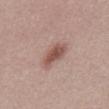Recorded during total-body skin imaging; not selected for excision or biopsy. About 4.5 mm across. A male patient in their 30s. A roughly 15 mm field-of-view crop from a total-body skin photograph. Automated tile analysis of the lesion measured a lesion area of about 7 mm², a shape eccentricity near 0.85, and two-axis asymmetry of about 0.25. It also reported border irregularity of about 3 on a 0–10 scale, a within-lesion color-variation index near 3.5/10, and a peripheral color-asymmetry measure near 1. The tile uses white-light illumination.